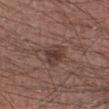No biopsy was performed on this lesion — it was imaged during a full skin examination and was not determined to be concerning.
The total-body-photography lesion software estimated an average lesion color of about L≈38 a*≈18 b*≈20 (CIELAB), a lesion–skin lightness drop of about 9, and a lesion-to-skin contrast of about 7.5 (normalized; higher = more distinct). It also reported border irregularity of about 2.5 on a 0–10 scale, internal color variation of about 4 on a 0–10 scale, and radial color variation of about 1.
The patient is a male roughly 45 years of age.
A lesion tile, about 15 mm wide, cut from a 3D total-body photograph.
On the left lower leg.
Captured under white-light illumination.
The recorded lesion diameter is about 3 mm.